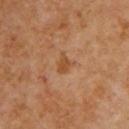notes = no biopsy performed (imaged during a skin exam) | lesion diameter = ≈2.5 mm | acquisition = total-body-photography crop, ~15 mm field of view | subject = male, aged around 60 | location = the chest | lighting = cross-polarized.